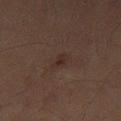Assessment: No biopsy was performed on this lesion — it was imaged during a full skin examination and was not determined to be concerning. Image and clinical context: A 15 mm close-up extracted from a 3D total-body photography capture. Located on the right thigh. Imaged with cross-polarized lighting. A male subject about 70 years old. The lesion's longest dimension is about 2.5 mm.